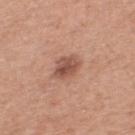Recorded during total-body skin imaging; not selected for excision or biopsy. A 15 mm close-up tile from a total-body photography series done for melanoma screening. Imaged with white-light lighting. The subject is a male aged 53 to 57. The lesion is on the upper back. Longest diameter approximately 3.5 mm.